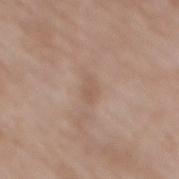{
  "biopsy_status": "not biopsied; imaged during a skin examination",
  "image": {
    "source": "total-body photography crop",
    "field_of_view_mm": 15
  },
  "lesion_size": {
    "long_diameter_mm_approx": 2.5
  },
  "lighting": "white-light",
  "patient": {
    "sex": "female",
    "age_approx": 75
  },
  "automated_metrics": {
    "area_mm2_approx": 3.0,
    "shape_asymmetry": 0.3,
    "cielab_L": 56,
    "cielab_a": 17,
    "cielab_b": 27,
    "vs_skin_darker_L": 6.0,
    "vs_skin_contrast_norm": 4.5,
    "border_irregularity_0_10": 3.0,
    "color_variation_0_10": 1.0,
    "peripheral_color_asymmetry": 0.5,
    "nevus_likeness_0_100": 0,
    "lesion_detection_confidence_0_100": 100
  },
  "site": "back"
}